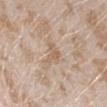{
  "biopsy_status": "not biopsied; imaged during a skin examination",
  "patient": {
    "sex": "female",
    "age_approx": 25
  },
  "lesion_size": {
    "long_diameter_mm_approx": 3.0
  },
  "site": "left forearm",
  "automated_metrics": {
    "area_mm2_approx": 3.5,
    "eccentricity": 0.7,
    "shape_asymmetry": 0.55,
    "cielab_L": 61,
    "cielab_a": 15,
    "cielab_b": 30,
    "vs_skin_darker_L": 7.0
  },
  "image": {
    "source": "total-body photography crop",
    "field_of_view_mm": 15
  },
  "lighting": "white-light"
}